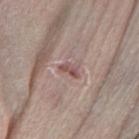<lesion>
<biopsy_status>not biopsied; imaged during a skin examination</biopsy_status>
<site>left forearm</site>
<lesion_size>
  <long_diameter_mm_approx>2.5</long_diameter_mm_approx>
</lesion_size>
<image>
  <source>total-body photography crop</source>
  <field_of_view_mm>15</field_of_view_mm>
</image>
<patient>
  <sex>male</sex>
  <age_approx>80</age_approx>
</patient>
<lighting>white-light</lighting>
</lesion>